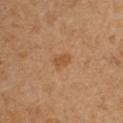workup = no biopsy performed (imaged during a skin exam).